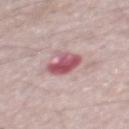Captured during whole-body skin photography for melanoma surveillance; the lesion was not biopsied.
A male subject approximately 50 years of age.
The lesion is on the mid back.
Automated image analysis of the tile measured an area of roughly 7.5 mm² and a symmetry-axis asymmetry near 0.2. The software also gave a mean CIELAB color near L≈54 a*≈29 b*≈17, about 15 CIELAB-L* units darker than the surrounding skin, and a normalized border contrast of about 10. The software also gave border irregularity of about 2.5 on a 0–10 scale, a within-lesion color-variation index near 6.5/10, and peripheral color asymmetry of about 2. The analysis additionally found a nevus-likeness score of about 0/100 and a detector confidence of about 100 out of 100 that the crop contains a lesion.
About 3.5 mm across.
A roughly 15 mm field-of-view crop from a total-body skin photograph.
Imaged with white-light lighting.